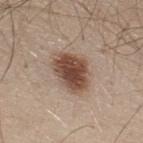The lesion was tiled from a total-body skin photograph and was not biopsied.
About 5 mm across.
This image is a 15 mm lesion crop taken from a total-body photograph.
Located on the upper back.
The tile uses white-light illumination.
Automated image analysis of the tile measured a footprint of about 14 mm² and a shape-asymmetry score of about 0.2 (0 = symmetric). The software also gave border irregularity of about 2 on a 0–10 scale and radial color variation of about 1.5.
A male patient in their 30s.